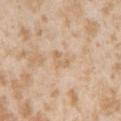subject: female, aged approximately 25
anatomic site: the arm
lighting: white-light illumination
image-analysis metrics: a border-irregularity index near 4.5/10, a within-lesion color-variation index near 0/10, and radial color variation of about 0
diameter: ~2.5 mm (longest diameter)
imaging modality: 15 mm crop, total-body photography